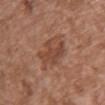The lesion was tiled from a total-body skin photograph and was not biopsied.
Cropped from a total-body skin-imaging series; the visible field is about 15 mm.
About 5 mm across.
A female patient approximately 75 years of age.
From the chest.
Captured under white-light illumination.